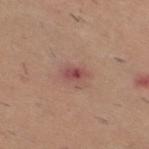{"biopsy_status": "not biopsied; imaged during a skin examination", "patient": {"sex": "male", "age_approx": 40}, "image": {"source": "total-body photography crop", "field_of_view_mm": 15}, "site": "right thigh"}